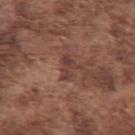The lesion was photographed on a routine skin check and not biopsied; there is no pathology result. Longest diameter approximately 3 mm. On the left upper arm. This image is a 15 mm lesion crop taken from a total-body photograph. Captured under white-light illumination. The total-body-photography lesion software estimated a lesion area of about 3 mm², an outline eccentricity of about 0.9 (0 = round, 1 = elongated), and two-axis asymmetry of about 0.5. It also reported a classifier nevus-likeness of about 0/100 and a lesion-detection confidence of about 90/100. The patient is a male aged approximately 75.